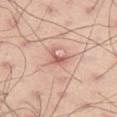Assessment:
Captured during whole-body skin photography for melanoma surveillance; the lesion was not biopsied.
Clinical summary:
From the left thigh. Approximately 2.5 mm at its widest. The subject is a male in their mid- to late 40s. A 15 mm close-up tile from a total-body photography series done for melanoma screening. The tile uses white-light illumination.